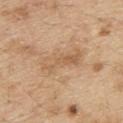Q: Was a biopsy performed?
A: imaged on a skin check; not biopsied
Q: What did automated image analysis measure?
A: roughly 8 lightness units darker than nearby skin and a lesion-to-skin contrast of about 5.5 (normalized; higher = more distinct); a border-irregularity rating of about 3.5/10, internal color variation of about 4.5 on a 0–10 scale, and radial color variation of about 1.5; an automated nevus-likeness rating near 0 out of 100
Q: How large is the lesion?
A: ~5.5 mm (longest diameter)
Q: Illumination type?
A: white-light
Q: Where on the body is the lesion?
A: the upper back
Q: Patient demographics?
A: male, aged approximately 70
Q: What is the imaging modality?
A: total-body-photography crop, ~15 mm field of view